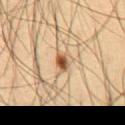| feature | finding |
|---|---|
| follow-up | total-body-photography surveillance lesion; no biopsy |